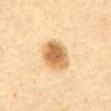Assessment:
Imaged during a routine full-body skin examination; the lesion was not biopsied and no histopathology is available.
Clinical summary:
A male patient, aged approximately 60. Captured under cross-polarized illumination. Cropped from a whole-body photographic skin survey; the tile spans about 15 mm. Approximately 4 mm at its widest. The lesion-visualizer software estimated a border-irregularity index near 1.5/10, internal color variation of about 5 on a 0–10 scale, and a peripheral color-asymmetry measure near 1.5. The software also gave a lesion-detection confidence of about 100/100. The lesion is on the abdomen.